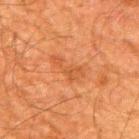Recorded during total-body skin imaging; not selected for excision or biopsy.
About 4.5 mm across.
A male subject about 60 years old.
The lesion is on the right upper arm.
A lesion tile, about 15 mm wide, cut from a 3D total-body photograph.
The tile uses cross-polarized illumination.